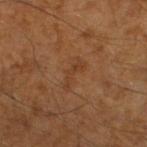Clinical summary:
Measured at roughly 3.5 mm in maximum diameter. A 15 mm crop from a total-body photograph taken for skin-cancer surveillance. The tile uses cross-polarized illumination. Automated image analysis of the tile measured a footprint of about 4 mm², an eccentricity of roughly 0.95, and a symmetry-axis asymmetry near 0.4. The analysis additionally found an average lesion color of about L≈35 a*≈19 b*≈30 (CIELAB), about 5 CIELAB-L* units darker than the surrounding skin, and a normalized border contrast of about 5. The software also gave a within-lesion color-variation index near 0/10. A male patient, in their 60s. On the left lower leg.